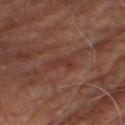Captured during whole-body skin photography for melanoma surveillance; the lesion was not biopsied. A region of skin cropped from a whole-body photographic capture, roughly 15 mm wide. Located on the left thigh. This is a cross-polarized tile. The subject is a male aged approximately 70. The recorded lesion diameter is about 2.5 mm.